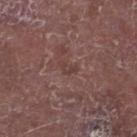{"biopsy_status": "not biopsied; imaged during a skin examination", "image": {"source": "total-body photography crop", "field_of_view_mm": 15}, "patient": {"sex": "male", "age_approx": 65}, "site": "right lower leg"}